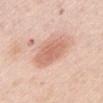This image is a 15 mm lesion crop taken from a total-body photograph. A female patient, aged 63 to 67. On the front of the torso. Captured under white-light illumination.